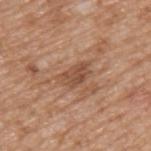notes: total-body-photography surveillance lesion; no biopsy | lesion size: ≈4 mm | site: the left upper arm | image source: 15 mm crop, total-body photography | subject: male, aged approximately 65.